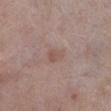biopsy_status: not biopsied; imaged during a skin examination
automated_metrics:
  eccentricity: 0.75
  cielab_L: 53
  cielab_a: 17
  cielab_b: 24
  vs_skin_darker_L: 6.0
  vs_skin_contrast_norm: 5.0
image:
  source: total-body photography crop
  field_of_view_mm: 15
patient:
  sex: male
  age_approx: 70
lesion_size:
  long_diameter_mm_approx: 2.5
site: left lower leg
lighting: white-light